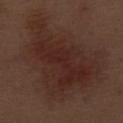follow-up: no biopsy performed (imaged during a skin exam).